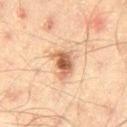follow-up=total-body-photography surveillance lesion; no biopsy
image=~15 mm tile from a whole-body skin photo
subject=male, aged 43 to 47
TBP lesion metrics=a border-irregularity rating of about 3.5/10, internal color variation of about 6 on a 0–10 scale, and radial color variation of about 1.5; an automated nevus-likeness rating near 90 out of 100 and lesion-presence confidence of about 100/100
body site=the left thigh
tile lighting=cross-polarized illumination